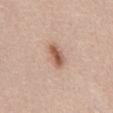Context: The lesion-visualizer software estimated a footprint of about 5.5 mm² and an outline eccentricity of about 0.85 (0 = round, 1 = elongated). The software also gave border irregularity of about 2.5 on a 0–10 scale and a color-variation rating of about 4/10. The lesion is on the abdomen. Cropped from a total-body skin-imaging series; the visible field is about 15 mm. This is a white-light tile. The patient is a female aged 28–32.